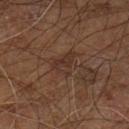The lesion was photographed on a routine skin check and not biopsied; there is no pathology result. From the right leg. Measured at roughly 3.5 mm in maximum diameter. A roughly 15 mm field-of-view crop from a total-body skin photograph. The total-body-photography lesion software estimated about 6 CIELAB-L* units darker than the surrounding skin and a lesion-to-skin contrast of about 6 (normalized; higher = more distinct). Imaged with cross-polarized lighting. A male patient aged approximately 60.